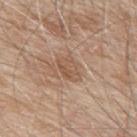Recorded during total-body skin imaging; not selected for excision or biopsy. The lesion is located on the back. The lesion-visualizer software estimated an area of roughly 5 mm² and a shape eccentricity near 0.8. It also reported an average lesion color of about L≈54 a*≈19 b*≈30 (CIELAB) and about 7 CIELAB-L* units darker than the surrounding skin. This is a white-light tile. A 15 mm close-up tile from a total-body photography series done for melanoma screening. Longest diameter approximately 3 mm. The patient is a male approximately 80 years of age.